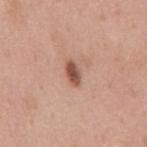- follow-up · imaged on a skin check; not biopsied
- lesion diameter · ≈3 mm
- site · the mid back
- illumination · white-light illumination
- automated metrics · a lesion–skin lightness drop of about 15 and a normalized border contrast of about 10; a border-irregularity rating of about 1.5/10, internal color variation of about 1.5 on a 0–10 scale, and radial color variation of about 0.5; a nevus-likeness score of about 100/100
- image source · 15 mm crop, total-body photography
- patient · male, aged 58–62